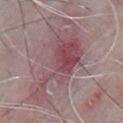This lesion was catalogued during total-body skin photography and was not selected for biopsy.
A male patient aged 63–67.
Located on the front of the torso.
A close-up tile cropped from a whole-body skin photograph, about 15 mm across.
Imaged with white-light lighting.